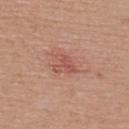Notes:
• follow-up: imaged on a skin check; not biopsied
• body site: the upper back
• subject: male, aged around 55
• acquisition: 15 mm crop, total-body photography